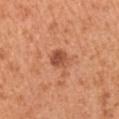image:
  source: total-body photography crop
  field_of_view_mm: 15
site: left upper arm
patient:
  sex: male
  age_approx: 55
lighting: white-light
lesion_size:
  long_diameter_mm_approx: 2.5